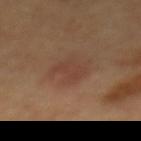Notes:
* biopsy status: no biopsy performed (imaged during a skin exam)
* patient: female, aged 58–62
* site: the mid back
* imaging modality: ~15 mm tile from a whole-body skin photo